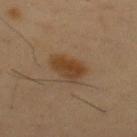biopsy status=catalogued during a skin exam; not biopsied
automated lesion analysis=a footprint of about 8.5 mm² and a symmetry-axis asymmetry near 0.3; a border-irregularity index near 3/10, a within-lesion color-variation index near 3.5/10, and radial color variation of about 1; a nevus-likeness score of about 100/100 and a detector confidence of about 100 out of 100 that the crop contains a lesion
lesion diameter=about 4 mm
subject=male, aged 38 to 42
image=total-body-photography crop, ~15 mm field of view
illumination=cross-polarized illumination
body site=the upper back